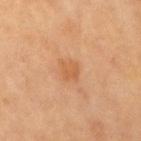<tbp_lesion>
<biopsy_status>not biopsied; imaged during a skin examination</biopsy_status>
<lesion_size>
  <long_diameter_mm_approx>2.5</long_diameter_mm_approx>
</lesion_size>
<site>right upper arm</site>
<patient>
  <sex>female</sex>
  <age_approx>65</age_approx>
</patient>
<lighting>cross-polarized</lighting>
<image>
  <source>total-body photography crop</source>
  <field_of_view_mm>15</field_of_view_mm>
</image>
</tbp_lesion>